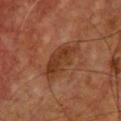<tbp_lesion>
<biopsy_status>not biopsied; imaged during a skin examination</biopsy_status>
<lighting>cross-polarized</lighting>
<image>
  <source>total-body photography crop</source>
  <field_of_view_mm>15</field_of_view_mm>
</image>
<lesion_size>
  <long_diameter_mm_approx>6.0</long_diameter_mm_approx>
</lesion_size>
<site>chest</site>
<patient>
  <sex>male</sex>
  <age_approx>60</age_approx>
</patient>
<automated_metrics>
  <area_mm2_approx>11.0</area_mm2_approx>
  <eccentricity>0.9</eccentricity>
  <shape_asymmetry>0.3</shape_asymmetry>
</automated_metrics>
</tbp_lesion>